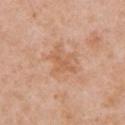Assessment: Imaged during a routine full-body skin examination; the lesion was not biopsied and no histopathology is available. Context: The lesion is located on the arm. This is a white-light tile. A lesion tile, about 15 mm wide, cut from a 3D total-body photograph. The subject is a female about 40 years old.